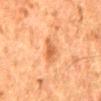This lesion was catalogued during total-body skin photography and was not selected for biopsy. A male subject aged 78 to 82. About 3 mm across. This is a cross-polarized tile. Automated tile analysis of the lesion measured a footprint of about 4.5 mm², an eccentricity of roughly 0.75, and a shape-asymmetry score of about 0.35 (0 = symmetric). The analysis additionally found a mean CIELAB color near L≈49 a*≈24 b*≈36, about 9 CIELAB-L* units darker than the surrounding skin, and a normalized border contrast of about 6.5. The software also gave internal color variation of about 2.5 on a 0–10 scale. The software also gave an automated nevus-likeness rating near 35 out of 100 and a lesion-detection confidence of about 100/100. Cropped from a total-body skin-imaging series; the visible field is about 15 mm. The lesion is located on the back.